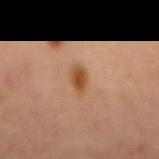Case summary:
– workup — no biopsy performed (imaged during a skin exam)
– automated metrics — a border-irregularity rating of about 2.5/10 and a peripheral color-asymmetry measure near 0.5; an automated nevus-likeness rating near 95 out of 100 and a detector confidence of about 100 out of 100 that the crop contains a lesion
– size — ~3.5 mm (longest diameter)
– subject — female
– lighting — cross-polarized
– body site — the abdomen
– acquisition — total-body-photography crop, ~15 mm field of view